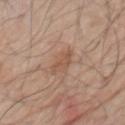| field | value |
|---|---|
| workup | total-body-photography surveillance lesion; no biopsy |
| image | 15 mm crop, total-body photography |
| subject | male, roughly 80 years of age |
| lesion diameter | about 4.5 mm |
| location | the chest |
| illumination | white-light illumination |